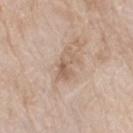Part of a total-body skin-imaging series; this lesion was reviewed on a skin check and was not flagged for biopsy.
An algorithmic analysis of the crop reported a lesion area of about 5.5 mm². The analysis additionally found a color-variation rating of about 5/10 and radial color variation of about 1.5. It also reported an automated nevus-likeness rating near 0 out of 100 and a lesion-detection confidence of about 100/100.
Located on the right upper arm.
This is a white-light tile.
Approximately 4 mm at its widest.
The patient is a female roughly 75 years of age.
A lesion tile, about 15 mm wide, cut from a 3D total-body photograph.